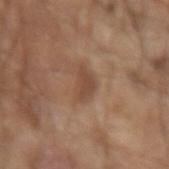Assessment:
The lesion was tiled from a total-body skin photograph and was not biopsied.
Background:
A male subject, aged approximately 80. The tile uses white-light illumination. A 15 mm crop from a total-body photograph taken for skin-cancer surveillance. Located on the left forearm. About 3.5 mm across. An algorithmic analysis of the crop reported internal color variation of about 1.5 on a 0–10 scale.